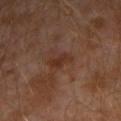Imaged during a routine full-body skin examination; the lesion was not biopsied and no histopathology is available. Located on the left arm. A 15 mm close-up extracted from a 3D total-body photography capture. The subject is a male roughly 30 years of age.